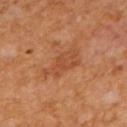Captured during whole-body skin photography for melanoma surveillance; the lesion was not biopsied. The tile uses cross-polarized illumination. Cropped from a whole-body photographic skin survey; the tile spans about 15 mm. A male subject, aged around 65. From the mid back.